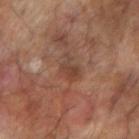The lesion was photographed on a routine skin check and not biopsied; there is no pathology result.
Automated image analysis of the tile measured a lesion color around L≈40 a*≈19 b*≈27 in CIELAB, roughly 7 lightness units darker than nearby skin, and a lesion-to-skin contrast of about 6 (normalized; higher = more distinct). The analysis additionally found border irregularity of about 4.5 on a 0–10 scale and internal color variation of about 3 on a 0–10 scale. And it measured a detector confidence of about 100 out of 100 that the crop contains a lesion.
A male subject aged approximately 65.
About 3.5 mm across.
A roughly 15 mm field-of-view crop from a total-body skin photograph.
Imaged with cross-polarized lighting.
From the right forearm.